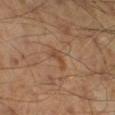Assessment: Imaged during a routine full-body skin examination; the lesion was not biopsied and no histopathology is available. Context: The subject is a male in their mid-40s. A 15 mm close-up tile from a total-body photography series done for melanoma screening. On the right lower leg.